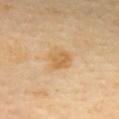Findings:
- size — ≈3 mm
- automated metrics — border irregularity of about 2 on a 0–10 scale, a color-variation rating of about 2.5/10, and a peripheral color-asymmetry measure near 1
- anatomic site — the mid back
- tile lighting — cross-polarized illumination
- image source — total-body-photography crop, ~15 mm field of view
- patient — female, aged approximately 60